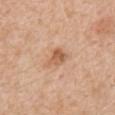The lesion was tiled from a total-body skin photograph and was not biopsied.
The patient is a male about 60 years old.
A close-up tile cropped from a whole-body skin photograph, about 15 mm across.
Automated tile analysis of the lesion measured a shape eccentricity near 0.6 and two-axis asymmetry of about 0.3. It also reported a border-irregularity rating of about 2.5/10, a within-lesion color-variation index near 3.5/10, and peripheral color asymmetry of about 1.
About 2.5 mm across.
This is a white-light tile.
The lesion is on the right upper arm.